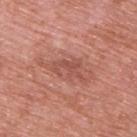No biopsy was performed on this lesion — it was imaged during a full skin examination and was not determined to be concerning. Measured at roughly 3.5 mm in maximum diameter. From the upper back. A region of skin cropped from a whole-body photographic capture, roughly 15 mm wide. The tile uses white-light illumination. The subject is a male roughly 70 years of age.